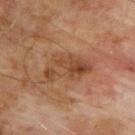Case summary:
– biopsy status · catalogued during a skin exam; not biopsied
– subject · male, aged approximately 65
– image source · ~15 mm crop, total-body skin-cancer survey
– illumination · cross-polarized
– lesion diameter · ~6 mm (longest diameter)
– body site · the back
– automated lesion analysis · a classifier nevus-likeness of about 0/100 and lesion-presence confidence of about 100/100